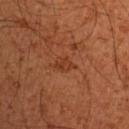Captured under cross-polarized illumination. The lesion is located on the right upper arm. A 15 mm crop from a total-body photograph taken for skin-cancer surveillance. The patient is a male about 50 years old. An algorithmic analysis of the crop reported an area of roughly 3.5 mm², an eccentricity of roughly 0.8, and two-axis asymmetry of about 0.35. The software also gave a lesion–skin lightness drop of about 6 and a lesion-to-skin contrast of about 5.5 (normalized; higher = more distinct). It also reported lesion-presence confidence of about 100/100.